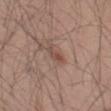Impression:
No biopsy was performed on this lesion — it was imaged during a full skin examination and was not determined to be concerning.
Clinical summary:
On the left thigh. Cropped from a whole-body photographic skin survey; the tile spans about 15 mm. The subject is a male aged around 45.